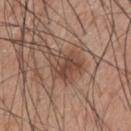follow-up: total-body-photography surveillance lesion; no biopsy
lesion diameter: ~4.5 mm (longest diameter)
image source: ~15 mm tile from a whole-body skin photo
patient: male, in their 20s
site: the upper back
illumination: white-light illumination